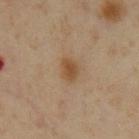| feature | finding |
|---|---|
| workup | imaged on a skin check; not biopsied |
| imaging modality | 15 mm crop, total-body photography |
| patient | male, aged approximately 60 |
| body site | the front of the torso |
| lighting | cross-polarized illumination |
| lesion diameter | about 2.5 mm |